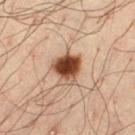Acquisition and patient details:
The lesion is located on the leg. A 15 mm crop from a total-body photograph taken for skin-cancer surveillance. A male patient, in their mid- to late 30s. An algorithmic analysis of the crop reported an average lesion color of about L≈43 a*≈21 b*≈30 (CIELAB), about 20 CIELAB-L* units darker than the surrounding skin, and a lesion-to-skin contrast of about 14 (normalized; higher = more distinct). Measured at roughly 3.5 mm in maximum diameter.